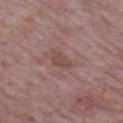Case summary:
• notes — total-body-photography surveillance lesion; no biopsy
• site — the right upper arm
• patient — male, approximately 65 years of age
• automated lesion analysis — a lesion color around L≈46 a*≈20 b*≈23 in CIELAB, a lesion–skin lightness drop of about 7, and a normalized border contrast of about 6.5; a within-lesion color-variation index near 1/10 and a peripheral color-asymmetry measure near 0.5
• imaging modality — ~15 mm crop, total-body skin-cancer survey
• diameter — about 2.5 mm